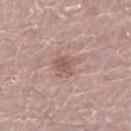<record>
<biopsy_status>not biopsied; imaged during a skin examination</biopsy_status>
<lighting>white-light</lighting>
<patient>
  <sex>male</sex>
  <age_approx>70</age_approx>
</patient>
<site>leg</site>
<automated_metrics>
  <area_mm2_approx>7.0</area_mm2_approx>
  <eccentricity>0.6</eccentricity>
  <shape_asymmetry>0.25</shape_asymmetry>
  <cielab_L>57</cielab_L>
  <cielab_a>18</cielab_a>
  <cielab_b>25</cielab_b>
</automated_metrics>
<image>
  <source>total-body photography crop</source>
  <field_of_view_mm>15</field_of_view_mm>
</image>
<lesion_size>
  <long_diameter_mm_approx>3.5</long_diameter_mm_approx>
</lesion_size>
</record>